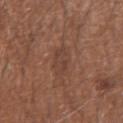| field | value |
|---|---|
| workup | imaged on a skin check; not biopsied |
| image source | total-body-photography crop, ~15 mm field of view |
| subject | male, about 70 years old |
| image-analysis metrics | a footprint of about 6 mm² and an eccentricity of roughly 0.7; a mean CIELAB color near L≈41 a*≈20 b*≈26 and about 5 CIELAB-L* units darker than the surrounding skin; a lesion-detection confidence of about 100/100 |
| anatomic site | the left forearm |
| tile lighting | white-light |